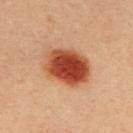biopsy status = no biopsy performed (imaged during a skin exam) | TBP lesion metrics = a mean CIELAB color near L≈48 a*≈30 b*≈36 and a normalized border contrast of about 12.5; border irregularity of about 1.5 on a 0–10 scale, internal color variation of about 7.5 on a 0–10 scale, and a peripheral color-asymmetry measure near 2; a classifier nevus-likeness of about 100/100 | location = the upper back | illumination = cross-polarized illumination | imaging modality = ~15 mm crop, total-body skin-cancer survey | patient = female, approximately 35 years of age | lesion diameter = ≈5.5 mm.